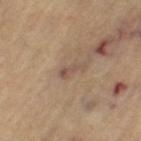Notes:
– workup: total-body-photography surveillance lesion; no biopsy
– lesion size: about 3 mm
– anatomic site: the left thigh
– acquisition: ~15 mm tile from a whole-body skin photo
– subject: female, approximately 65 years of age